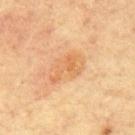workup: catalogued during a skin exam; not biopsied
anatomic site: the mid back
diameter: ~4.5 mm (longest diameter)
automated metrics: a footprint of about 8.5 mm² and an outline eccentricity of about 0.85 (0 = round, 1 = elongated); a border-irregularity index near 5/10, a within-lesion color-variation index near 3.5/10, and peripheral color asymmetry of about 1
image source: ~15 mm crop, total-body skin-cancer survey
patient: male, aged around 65
illumination: cross-polarized